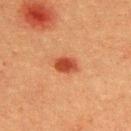Part of a total-body skin-imaging series; this lesion was reviewed on a skin check and was not flagged for biopsy.